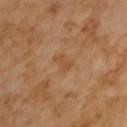Q: Is there a histopathology result?
A: catalogued during a skin exam; not biopsied
Q: What kind of image is this?
A: ~15 mm crop, total-body skin-cancer survey
Q: Automated lesion metrics?
A: a lesion–skin lightness drop of about 5 and a lesion-to-skin contrast of about 5 (normalized; higher = more distinct); a classifier nevus-likeness of about 0/100 and a lesion-detection confidence of about 100/100
Q: Who is the patient?
A: male, approximately 65 years of age
Q: Illumination type?
A: cross-polarized
Q: Lesion size?
A: ≈3 mm